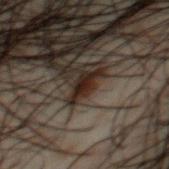{"biopsy_status": "not biopsied; imaged during a skin examination", "site": "chest", "automated_metrics": {"nevus_likeness_0_100": 100, "lesion_detection_confidence_0_100": 90}, "lighting": "cross-polarized", "patient": {"sex": "male", "age_approx": 50}, "image": {"source": "total-body photography crop", "field_of_view_mm": 15}}